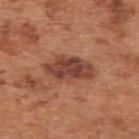{"biopsy_status": "not biopsied; imaged during a skin examination", "site": "upper back", "patient": {"sex": "male", "age_approx": 65}, "image": {"source": "total-body photography crop", "field_of_view_mm": 15}, "lighting": "white-light"}